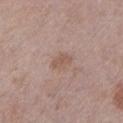| feature | finding |
|---|---|
| biopsy status | no biopsy performed (imaged during a skin exam) |
| image-analysis metrics | an eccentricity of roughly 0.85 and two-axis asymmetry of about 0.25; a lesion-to-skin contrast of about 6 (normalized; higher = more distinct); a border-irregularity index near 3/10 and radial color variation of about 0.5; a detector confidence of about 100 out of 100 that the crop contains a lesion |
| size | about 3 mm |
| body site | the left lower leg |
| patient | female, approximately 60 years of age |
| imaging modality | ~15 mm tile from a whole-body skin photo |